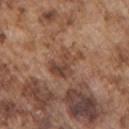workup: total-body-photography surveillance lesion; no biopsy
image source: total-body-photography crop, ~15 mm field of view
lesion size: ~4 mm (longest diameter)
body site: the left upper arm
tile lighting: white-light illumination
patient: male, aged around 75